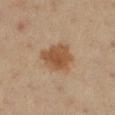| feature | finding |
|---|---|
| biopsy status | no biopsy performed (imaged during a skin exam) |
| subject | female, aged 48–52 |
| body site | the left lower leg |
| illumination | cross-polarized |
| image source | 15 mm crop, total-body photography |
| diameter | ≈4.5 mm |